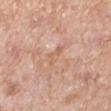<case>
<biopsy_status>not biopsied; imaged during a skin examination</biopsy_status>
<lesion_size>
  <long_diameter_mm_approx>2.5</long_diameter_mm_approx>
</lesion_size>
<patient>
  <sex>female</sex>
  <age_approx>70</age_approx>
</patient>
<lighting>white-light</lighting>
<site>left lower leg</site>
<image>
  <source>total-body photography crop</source>
  <field_of_view_mm>15</field_of_view_mm>
</image>
</case>